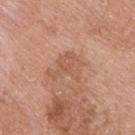Findings:
– workup · imaged on a skin check; not biopsied
– lighting · white-light
– subject · male, about 75 years old
– imaging modality · ~15 mm tile from a whole-body skin photo
– size · ≈4.5 mm
– location · the upper back